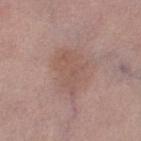A female patient, roughly 40 years of age. Cropped from a total-body skin-imaging series; the visible field is about 15 mm. Captured under white-light illumination. Measured at roughly 5.5 mm in maximum diameter. The lesion is located on the left lower leg.